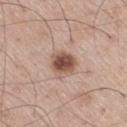Assessment:
This lesion was catalogued during total-body skin photography and was not selected for biopsy.
Clinical summary:
A male patient in their 60s. A region of skin cropped from a whole-body photographic capture, roughly 15 mm wide. Located on the right thigh. About 3.5 mm across. The lesion-visualizer software estimated a footprint of about 7.5 mm² and a shape-asymmetry score of about 0.2 (0 = symmetric). It also reported a mean CIELAB color near L≈52 a*≈20 b*≈27, about 15 CIELAB-L* units darker than the surrounding skin, and a normalized border contrast of about 10.5. It also reported a border-irregularity rating of about 1.5/10 and radial color variation of about 1.5.